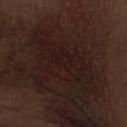{"biopsy_status": "not biopsied; imaged during a skin examination", "image": {"source": "total-body photography crop", "field_of_view_mm": 15}, "lesion_size": {"long_diameter_mm_approx": 9.0}, "lighting": "white-light", "patient": {"sex": "male", "age_approx": 70}, "site": "abdomen"}